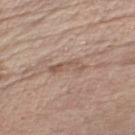Impression: Imaged during a routine full-body skin examination; the lesion was not biopsied and no histopathology is available. Acquisition and patient details: The lesion's longest dimension is about 4.5 mm. Automated image analysis of the tile measured a lesion area of about 5 mm², an outline eccentricity of about 0.95 (0 = round, 1 = elongated), and two-axis asymmetry of about 0.45. The software also gave a border-irregularity index near 6/10, internal color variation of about 1 on a 0–10 scale, and peripheral color asymmetry of about 0.5. The software also gave a classifier nevus-likeness of about 0/100 and lesion-presence confidence of about 55/100. Located on the left lower leg. The tile uses white-light illumination. The subject is a male aged around 70. A region of skin cropped from a whole-body photographic capture, roughly 15 mm wide.